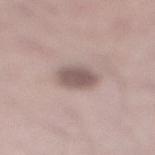| field | value |
|---|---|
| workup | no biopsy performed (imaged during a skin exam) |
| site | the right lower leg |
| automated lesion analysis | an area of roughly 7.5 mm², a shape eccentricity near 0.4, and a symmetry-axis asymmetry near 0.15; an average lesion color of about L≈54 a*≈15 b*≈19 (CIELAB), about 13 CIELAB-L* units darker than the surrounding skin, and a normalized border contrast of about 8.5 |
| lighting | white-light illumination |
| subject | male, about 35 years old |
| image source | total-body-photography crop, ~15 mm field of view |